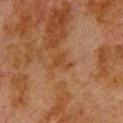Q: Was a biopsy performed?
A: total-body-photography surveillance lesion; no biopsy
Q: Illumination type?
A: cross-polarized illumination
Q: Lesion location?
A: the upper back
Q: What are the patient's age and sex?
A: male, about 80 years old
Q: What kind of image is this?
A: total-body-photography crop, ~15 mm field of view
Q: What did automated image analysis measure?
A: a lesion area of about 3 mm² and a shape eccentricity near 0.75; a lesion–skin lightness drop of about 5